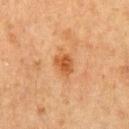The lesion was tiled from a total-body skin photograph and was not biopsied. A male patient aged 63–67. Automated image analysis of the tile measured an area of roughly 4 mm², an outline eccentricity of about 0.6 (0 = round, 1 = elongated), and two-axis asymmetry of about 0.35. The analysis additionally found border irregularity of about 3 on a 0–10 scale, a color-variation rating of about 2.5/10, and a peripheral color-asymmetry measure near 1. The analysis additionally found a classifier nevus-likeness of about 80/100 and lesion-presence confidence of about 100/100. From the mid back. Cropped from a whole-body photographic skin survey; the tile spans about 15 mm. Measured at roughly 2.5 mm in maximum diameter.